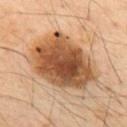Clinical impression: Part of a total-body skin-imaging series; this lesion was reviewed on a skin check and was not flagged for biopsy. Image and clinical context: A 15 mm crop from a total-body photograph taken for skin-cancer surveillance. On the mid back. Longest diameter approximately 8.5 mm. The subject is a male aged 48–52.